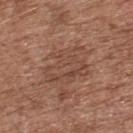No biopsy was performed on this lesion — it was imaged during a full skin examination and was not determined to be concerning.
The patient is a male aged around 75.
The lesion is located on the upper back.
Imaged with white-light lighting.
Cropped from a whole-body photographic skin survey; the tile spans about 15 mm.